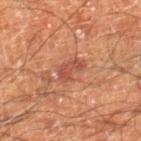Impression:
Recorded during total-body skin imaging; not selected for excision or biopsy.
Context:
Located on the leg. An algorithmic analysis of the crop reported an average lesion color of about L≈47 a*≈26 b*≈29 (CIELAB), roughly 9 lightness units darker than nearby skin, and a normalized border contrast of about 7. A region of skin cropped from a whole-body photographic capture, roughly 15 mm wide. About 3.5 mm across. A male patient aged 58–62. Imaged with cross-polarized lighting.